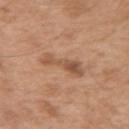notes = no biopsy performed (imaged during a skin exam) | lighting = white-light | acquisition = ~15 mm crop, total-body skin-cancer survey | patient = male, aged approximately 55 | location = the right upper arm | lesion diameter = ≈4.5 mm.